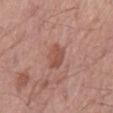Captured during whole-body skin photography for melanoma surveillance; the lesion was not biopsied.
A 15 mm close-up extracted from a 3D total-body photography capture.
The lesion is on the lower back.
The tile uses white-light illumination.
A male subject aged around 55.